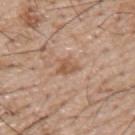Q: Was a biopsy performed?
A: imaged on a skin check; not biopsied
Q: Illumination type?
A: white-light
Q: How was this image acquired?
A: ~15 mm tile from a whole-body skin photo
Q: What is the lesion's diameter?
A: ≈3 mm
Q: Patient demographics?
A: male, approximately 55 years of age